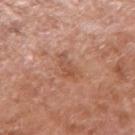{"biopsy_status": "not biopsied; imaged during a skin examination", "automated_metrics": {"border_irregularity_0_10": 5.0, "color_variation_0_10": 2.5, "peripheral_color_asymmetry": 0.5}, "site": "right upper arm", "image": {"source": "total-body photography crop", "field_of_view_mm": 15}, "patient": {"sex": "male", "age_approx": 65}, "lighting": "white-light", "lesion_size": {"long_diameter_mm_approx": 3.5}}